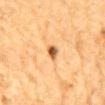Imaged during a routine full-body skin examination; the lesion was not biopsied and no histopathology is available. Located on the mid back. A lesion tile, about 15 mm wide, cut from a 3D total-body photograph. The tile uses cross-polarized illumination. The subject is a male in their mid- to late 80s or older. Measured at roughly 3 mm in maximum diameter.